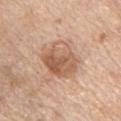follow-up: imaged on a skin check; not biopsied | size: ~4.5 mm (longest diameter) | location: the chest | TBP lesion metrics: a border-irregularity index near 2/10 and internal color variation of about 7.5 on a 0–10 scale; a classifier nevus-likeness of about 15/100 and a detector confidence of about 100 out of 100 that the crop contains a lesion | tile lighting: white-light illumination | acquisition: ~15 mm crop, total-body skin-cancer survey | subject: male, aged around 60.